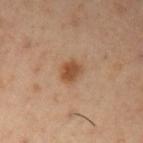Captured during whole-body skin photography for melanoma surveillance; the lesion was not biopsied. Imaged with cross-polarized lighting. A male subject, aged approximately 55. Cropped from a whole-body photographic skin survey; the tile spans about 15 mm. Measured at roughly 2.5 mm in maximum diameter. The lesion is on the left upper arm.